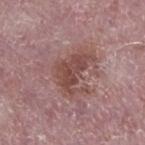Q: Is there a histopathology result?
A: imaged on a skin check; not biopsied
Q: Patient demographics?
A: male, aged 73 to 77
Q: Automated lesion metrics?
A: an average lesion color of about L≈47 a*≈22 b*≈22 (CIELAB), a lesion–skin lightness drop of about 9, and a lesion-to-skin contrast of about 7.5 (normalized; higher = more distinct); border irregularity of about 7.5 on a 0–10 scale and peripheral color asymmetry of about 1
Q: What lighting was used for the tile?
A: white-light
Q: What is the imaging modality?
A: ~15 mm crop, total-body skin-cancer survey
Q: What is the lesion's diameter?
A: ~5 mm (longest diameter)
Q: Lesion location?
A: the left lower leg